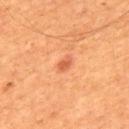biopsy status: total-body-photography surveillance lesion; no biopsy
lighting: cross-polarized
patient: male, approximately 55 years of age
size: ~2 mm (longest diameter)
automated metrics: border irregularity of about 3 on a 0–10 scale and a within-lesion color-variation index near 0.5/10; an automated nevus-likeness rating near 80 out of 100
location: the upper back
image: total-body-photography crop, ~15 mm field of view